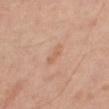Impression: Recorded during total-body skin imaging; not selected for excision or biopsy. Acquisition and patient details: This image is a 15 mm lesion crop taken from a total-body photograph. An algorithmic analysis of the crop reported a lesion area of about 3.5 mm², a shape eccentricity near 0.95, and two-axis asymmetry of about 0.3. The software also gave an automated nevus-likeness rating near 0 out of 100 and a lesion-detection confidence of about 100/100. A male patient aged around 65. Located on the leg.